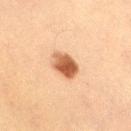Assessment:
Part of a total-body skin-imaging series; this lesion was reviewed on a skin check and was not flagged for biopsy.
Acquisition and patient details:
A male subject roughly 60 years of age. This is a cross-polarized tile. Cropped from a whole-body photographic skin survey; the tile spans about 15 mm. The total-body-photography lesion software estimated a lesion area of about 7 mm², a shape eccentricity near 0.6, and a shape-asymmetry score of about 0.2 (0 = symmetric). The software also gave an average lesion color of about L≈49 a*≈22 b*≈33 (CIELAB), roughly 16 lightness units darker than nearby skin, and a lesion-to-skin contrast of about 11 (normalized; higher = more distinct). The analysis additionally found an automated nevus-likeness rating near 100 out of 100 and a detector confidence of about 100 out of 100 that the crop contains a lesion. Longest diameter approximately 3.5 mm. On the right thigh.